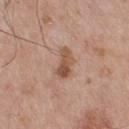Captured during whole-body skin photography for melanoma surveillance; the lesion was not biopsied. The lesion is located on the upper back. A male patient, in their mid- to late 50s. Captured under white-light illumination. Cropped from a total-body skin-imaging series; the visible field is about 15 mm. The lesion's longest dimension is about 3.5 mm. Automated tile analysis of the lesion measured an average lesion color of about L≈53 a*≈20 b*≈30 (CIELAB) and a normalized lesion–skin contrast near 8. The analysis additionally found border irregularity of about 4 on a 0–10 scale, a color-variation rating of about 5.5/10, and peripheral color asymmetry of about 2. The software also gave a detector confidence of about 100 out of 100 that the crop contains a lesion.